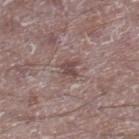Clinical summary:
The lesion is on the right lower leg. Longest diameter approximately 2.5 mm. Automated image analysis of the tile measured an average lesion color of about L≈47 a*≈17 b*≈19 (CIELAB) and a normalized border contrast of about 6.5. The software also gave an automated nevus-likeness rating near 0 out of 100 and a lesion-detection confidence of about 100/100. Captured under white-light illumination. A male patient, aged approximately 50. This image is a 15 mm lesion crop taken from a total-body photograph.